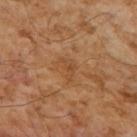Assessment:
No biopsy was performed on this lesion — it was imaged during a full skin examination and was not determined to be concerning.